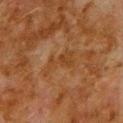Q: Is there a histopathology result?
A: imaged on a skin check; not biopsied
Q: What kind of image is this?
A: 15 mm crop, total-body photography
Q: What is the anatomic site?
A: the upper back
Q: Lesion size?
A: ≈4.5 mm
Q: Automated lesion metrics?
A: an area of roughly 7 mm², an outline eccentricity of about 0.9 (0 = round, 1 = elongated), and a shape-asymmetry score of about 0.5 (0 = symmetric); an average lesion color of about L≈33 a*≈18 b*≈31 (CIELAB) and a normalized lesion–skin contrast near 5.5; border irregularity of about 7.5 on a 0–10 scale, a within-lesion color-variation index near 1.5/10, and peripheral color asymmetry of about 0.5; a classifier nevus-likeness of about 0/100 and a lesion-detection confidence of about 85/100
Q: Patient demographics?
A: male, about 80 years old
Q: How was the tile lit?
A: cross-polarized illumination